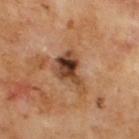follow-up: no biopsy performed (imaged during a skin exam)
site: the upper back
automated metrics: a mean CIELAB color near L≈42 a*≈21 b*≈31, about 14 CIELAB-L* units darker than the surrounding skin, and a lesion-to-skin contrast of about 10.5 (normalized; higher = more distinct)
lesion diameter: about 5 mm
tile lighting: cross-polarized
patient: male, about 70 years old
imaging modality: ~15 mm tile from a whole-body skin photo